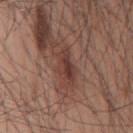follow-up: no biopsy performed (imaged during a skin exam)